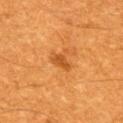| feature | finding |
|---|---|
| workup | total-body-photography surveillance lesion; no biopsy |